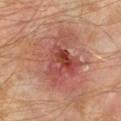Notes:
• biopsy status: catalogued during a skin exam; not biopsied
• tile lighting: cross-polarized illumination
• automated lesion analysis: a mean CIELAB color near L≈48 a*≈25 b*≈26, about 9 CIELAB-L* units darker than the surrounding skin, and a normalized lesion–skin contrast near 6.5; a border-irregularity rating of about 4.5/10, internal color variation of about 9 on a 0–10 scale, and radial color variation of about 2.5
• lesion size: ~7.5 mm (longest diameter)
• location: the left lower leg
• imaging modality: ~15 mm crop, total-body skin-cancer survey
• patient: male, aged 58–62